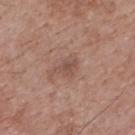Recorded during total-body skin imaging; not selected for excision or biopsy. The subject is a male in their 70s. Longest diameter approximately 3 mm. On the upper back. A 15 mm close-up tile from a total-body photography series done for melanoma screening. The tile uses white-light illumination.